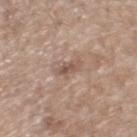The lesion was photographed on a routine skin check and not biopsied; there is no pathology result.
A 15 mm close-up extracted from a 3D total-body photography capture.
Approximately 3 mm at its widest.
A male subject, aged approximately 70.
From the head or neck.